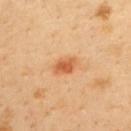| feature | finding |
|---|---|
| patient | male, about 40 years old |
| automated lesion analysis | about 12 CIELAB-L* units darker than the surrounding skin and a lesion-to-skin contrast of about 8 (normalized; higher = more distinct); a classifier nevus-likeness of about 95/100 and lesion-presence confidence of about 100/100 |
| size | about 3 mm |
| acquisition | ~15 mm crop, total-body skin-cancer survey |
| body site | the upper back |
| tile lighting | cross-polarized |